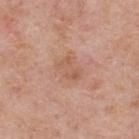{
  "biopsy_status": "not biopsied; imaged during a skin examination",
  "site": "back",
  "patient": {
    "sex": "male",
    "age_approx": 75
  },
  "lighting": "white-light",
  "lesion_size": {
    "long_diameter_mm_approx": 3.0
  },
  "automated_metrics": {
    "area_mm2_approx": 4.5,
    "eccentricity": 0.8,
    "nevus_likeness_0_100": 0,
    "lesion_detection_confidence_0_100": 100
  },
  "image": {
    "source": "total-body photography crop",
    "field_of_view_mm": 15
  }
}